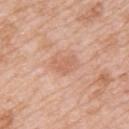Clinical impression: The lesion was tiled from a total-body skin photograph and was not biopsied. Context: A 15 mm close-up tile from a total-body photography series done for melanoma screening. A female patient aged 43 to 47. This is a white-light tile. On the back.